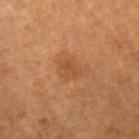<lesion>
  <biopsy_status>not biopsied; imaged during a skin examination</biopsy_status>
  <site>left upper arm</site>
  <patient>
    <sex>male</sex>
    <age_approx>75</age_approx>
  </patient>
  <automated_metrics>
    <area_mm2_approx>4.5</area_mm2_approx>
    <eccentricity>0.5</eccentricity>
    <shape_asymmetry>0.3</shape_asymmetry>
    <border_irregularity_0_10>3.0</border_irregularity_0_10>
    <color_variation_0_10>1.5</color_variation_0_10>
    <peripheral_color_asymmetry>0.5</peripheral_color_asymmetry>
    <lesion_detection_confidence_0_100>100</lesion_detection_confidence_0_100>
  </automated_metrics>
  <image>
    <source>total-body photography crop</source>
    <field_of_view_mm>15</field_of_view_mm>
  </image>
  <lighting>cross-polarized</lighting>
  <lesion_size>
    <long_diameter_mm_approx>2.5</long_diameter_mm_approx>
  </lesion_size>
</lesion>